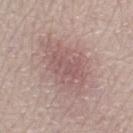follow-up — total-body-photography surveillance lesion; no biopsy | illumination — white-light illumination | acquisition — total-body-photography crop, ~15 mm field of view | automated metrics — a lesion color around L≈57 a*≈19 b*≈20 in CIELAB, roughly 8 lightness units darker than nearby skin, and a normalized lesion–skin contrast near 5.5; border irregularity of about 4 on a 0–10 scale, a within-lesion color-variation index near 3.5/10, and radial color variation of about 1 | size — about 7.5 mm | location — the right lower leg | subject — female, approximately 60 years of age.